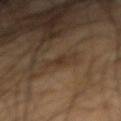Clinical impression:
No biopsy was performed on this lesion — it was imaged during a full skin examination and was not determined to be concerning.
Clinical summary:
Measured at roughly 3 mm in maximum diameter. The total-body-photography lesion software estimated an outline eccentricity of about 0.8 (0 = round, 1 = elongated). The analysis additionally found an average lesion color of about L≈33 a*≈14 b*≈26 (CIELAB), about 5 CIELAB-L* units darker than the surrounding skin, and a lesion-to-skin contrast of about 5.5 (normalized; higher = more distinct). The analysis additionally found a border-irregularity index near 3.5/10 and a color-variation rating of about 1.5/10. Cropped from a total-body skin-imaging series; the visible field is about 15 mm. This is a cross-polarized tile. A male subject in their mid- to late 60s. Located on the right forearm.